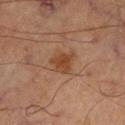follow-up: total-body-photography surveillance lesion; no biopsy | patient: male, approximately 65 years of age | lesion size: ≈3 mm | image source: ~15 mm tile from a whole-body skin photo | TBP lesion metrics: a lesion color around L≈34 a*≈18 b*≈27 in CIELAB, roughly 7 lightness units darker than nearby skin, and a lesion-to-skin contrast of about 7.5 (normalized; higher = more distinct); a border-irregularity rating of about 2.5/10, a within-lesion color-variation index near 2/10, and radial color variation of about 0.5; a nevus-likeness score of about 65/100 and a detector confidence of about 100 out of 100 that the crop contains a lesion | illumination: cross-polarized | location: the right lower leg.